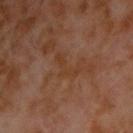Q: Was a biopsy performed?
A: catalogued during a skin exam; not biopsied
Q: What did automated image analysis measure?
A: an area of roughly 4.5 mm²; a lesion color around L≈35 a*≈19 b*≈29 in CIELAB, roughly 4 lightness units darker than nearby skin, and a normalized lesion–skin contrast near 5; a classifier nevus-likeness of about 0/100 and a lesion-detection confidence of about 95/100
Q: Who is the patient?
A: male, about 60 years old
Q: Where on the body is the lesion?
A: the upper back
Q: What lighting was used for the tile?
A: cross-polarized illumination
Q: What is the imaging modality?
A: total-body-photography crop, ~15 mm field of view